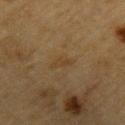Part of a total-body skin-imaging series; this lesion was reviewed on a skin check and was not flagged for biopsy.
A 15 mm crop from a total-body photograph taken for skin-cancer surveillance.
A male subject in their mid-80s or older.
The tile uses cross-polarized illumination.
On the left upper arm.
Measured at roughly 3 mm in maximum diameter.